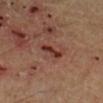- notes — total-body-photography surveillance lesion; no biopsy
- illumination — cross-polarized illumination
- image — ~15 mm tile from a whole-body skin photo
- anatomic site — the leg
- image-analysis metrics — an average lesion color of about L≈34 a*≈24 b*≈26 (CIELAB) and a normalized lesion–skin contrast near 9.5; a nevus-likeness score of about 0/100 and lesion-presence confidence of about 100/100
- patient — male, roughly 55 years of age
- size — about 3.5 mm